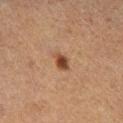Q: Was a biopsy performed?
A: catalogued during a skin exam; not biopsied
Q: How was this image acquired?
A: ~15 mm tile from a whole-body skin photo
Q: What lighting was used for the tile?
A: cross-polarized illumination
Q: What is the lesion's diameter?
A: ≈2.5 mm
Q: Patient demographics?
A: female, in their 40s
Q: What did automated image analysis measure?
A: a footprint of about 4 mm², a shape eccentricity near 0.75, and a shape-asymmetry score of about 0.2 (0 = symmetric); an average lesion color of about L≈38 a*≈19 b*≈28 (CIELAB), a lesion–skin lightness drop of about 11, and a lesion-to-skin contrast of about 9.5 (normalized; higher = more distinct); radial color variation of about 1; a nevus-likeness score of about 95/100 and a lesion-detection confidence of about 100/100
Q: Lesion location?
A: the right lower leg